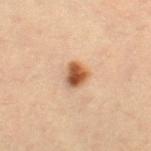The lesion was photographed on a routine skin check and not biopsied; there is no pathology result. A female patient, aged approximately 30. This image is a 15 mm lesion crop taken from a total-body photograph. The lesion is on the right thigh.